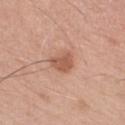No biopsy was performed on this lesion — it was imaged during a full skin examination and was not determined to be concerning.
A 15 mm close-up tile from a total-body photography series done for melanoma screening.
On the right forearm.
The tile uses white-light illumination.
About 2.5 mm across.
A female subject roughly 50 years of age.